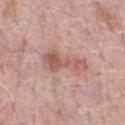Recorded during total-body skin imaging; not selected for excision or biopsy. The recorded lesion diameter is about 5.5 mm. The tile uses white-light illumination. Cropped from a total-body skin-imaging series; the visible field is about 15 mm. A male patient in their 70s. The lesion-visualizer software estimated border irregularity of about 6.5 on a 0–10 scale, a within-lesion color-variation index near 3.5/10, and radial color variation of about 1. The software also gave a nevus-likeness score of about 0/100 and a lesion-detection confidence of about 100/100. Located on the front of the torso.